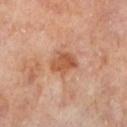Clinical summary: Approximately 3.5 mm at its widest. The patient is a male aged 63–67. The lesion is located on the left lower leg. A 15 mm close-up extracted from a 3D total-body photography capture. Captured under cross-polarized illumination.